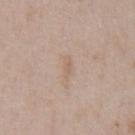Background: Located on the chest. This image is a 15 mm lesion crop taken from a total-body photograph. A male patient, in their 50s.